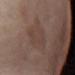imaging modality = total-body-photography crop, ~15 mm field of view; body site = the abdomen; subject = female, about 55 years old.